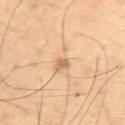* follow-up — imaged on a skin check; not biopsied
* lighting — cross-polarized
* image source — ~15 mm tile from a whole-body skin photo
* image-analysis metrics — a lesion area of about 6 mm², an eccentricity of roughly 0.75, and a shape-asymmetry score of about 0.5 (0 = symmetric); a lesion color around L≈69 a*≈18 b*≈37 in CIELAB, roughly 8 lightness units darker than nearby skin, and a normalized border contrast of about 5; a border-irregularity index near 5.5/10, internal color variation of about 3.5 on a 0–10 scale, and a peripheral color-asymmetry measure near 1; an automated nevus-likeness rating near 5 out of 100 and a lesion-detection confidence of about 100/100
* size — ~3.5 mm (longest diameter)
* subject — male, about 40 years old
* location — the upper back